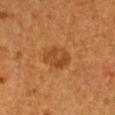The lesion was photographed on a routine skin check and not biopsied; there is no pathology result.
A region of skin cropped from a whole-body photographic capture, roughly 15 mm wide.
This is a cross-polarized tile.
A female subject, about 30 years old.
Located on the chest.
Longest diameter approximately 4 mm.
Automated image analysis of the tile measured a footprint of about 8 mm². The analysis additionally found a mean CIELAB color near L≈42 a*≈24 b*≈38, a lesion–skin lightness drop of about 8, and a normalized border contrast of about 6.5. The analysis additionally found a border-irregularity index near 2/10, internal color variation of about 3.5 on a 0–10 scale, and a peripheral color-asymmetry measure near 1.5. The software also gave a classifier nevus-likeness of about 85/100.